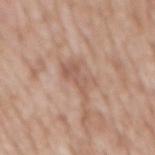{
  "biopsy_status": "not biopsied; imaged during a skin examination",
  "patient": {
    "sex": "male",
    "age_approx": 60
  },
  "site": "abdomen",
  "image": {
    "source": "total-body photography crop",
    "field_of_view_mm": 15
  }
}